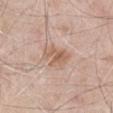Q: What is the lesion's diameter?
A: ≈3.5 mm
Q: What is the imaging modality?
A: ~15 mm tile from a whole-body skin photo
Q: What are the patient's age and sex?
A: male, aged 63 to 67
Q: Lesion location?
A: the right upper arm
Q: Automated lesion metrics?
A: a nevus-likeness score of about 45/100